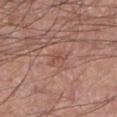Imaged during a routine full-body skin examination; the lesion was not biopsied and no histopathology is available. Automated image analysis of the tile measured a lesion color around L≈51 a*≈23 b*≈27 in CIELAB, about 6 CIELAB-L* units darker than the surrounding skin, and a normalized border contrast of about 4.5. And it measured a border-irregularity rating of about 4/10, internal color variation of about 2 on a 0–10 scale, and radial color variation of about 0.5. The software also gave a classifier nevus-likeness of about 0/100. Captured under white-light illumination. On the right lower leg. A 15 mm close-up tile from a total-body photography series done for melanoma screening. Approximately 2.5 mm at its widest. A male subject, aged around 45.